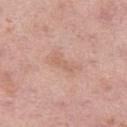biopsy status: no biopsy performed (imaged during a skin exam)
lighting: white-light illumination
acquisition: 15 mm crop, total-body photography
anatomic site: the left thigh
automated lesion analysis: an average lesion color of about L≈63 a*≈20 b*≈29 (CIELAB), roughly 6 lightness units darker than nearby skin, and a lesion-to-skin contrast of about 4.5 (normalized; higher = more distinct); a border-irregularity rating of about 5/10, internal color variation of about 1.5 on a 0–10 scale, and peripheral color asymmetry of about 0.5
subject: female, about 50 years old
diameter: ≈4.5 mm